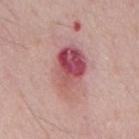biopsy status — total-body-photography surveillance lesion; no biopsy
lesion diameter — about 6 mm
subject — male, approximately 50 years of age
automated metrics — border irregularity of about 2.5 on a 0–10 scale, a color-variation rating of about 10/10, and radial color variation of about 5; a nevus-likeness score of about 0/100 and a lesion-detection confidence of about 100/100
illumination — white-light
location — the mid back
image source — ~15 mm tile from a whole-body skin photo